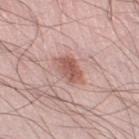Q: Is there a histopathology result?
A: no biopsy performed (imaged during a skin exam)
Q: Who is the patient?
A: male, about 25 years old
Q: What lighting was used for the tile?
A: white-light illumination
Q: What is the lesion's diameter?
A: ~3.5 mm (longest diameter)
Q: Automated lesion metrics?
A: border irregularity of about 2 on a 0–10 scale, internal color variation of about 3.5 on a 0–10 scale, and a peripheral color-asymmetry measure near 1; an automated nevus-likeness rating near 65 out of 100
Q: How was this image acquired?
A: total-body-photography crop, ~15 mm field of view
Q: What is the anatomic site?
A: the right thigh